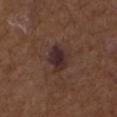Findings:
– workup: total-body-photography surveillance lesion; no biopsy
– acquisition: ~15 mm tile from a whole-body skin photo
– patient: male, aged 73–77
– lesion size: ~3.5 mm (longest diameter)
– lighting: white-light illumination
– anatomic site: the arm